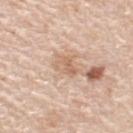Clinical impression:
Imaged during a routine full-body skin examination; the lesion was not biopsied and no histopathology is available.
Clinical summary:
A close-up tile cropped from a whole-body skin photograph, about 15 mm across. A female subject, roughly 50 years of age. From the right upper arm.